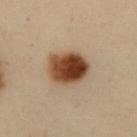Captured during whole-body skin photography for melanoma surveillance; the lesion was not biopsied. From the abdomen. A close-up tile cropped from a whole-body skin photograph, about 15 mm across. A female subject, aged around 50. Imaged with cross-polarized lighting. Measured at roughly 5 mm in maximum diameter. The lesion-visualizer software estimated a footprint of about 16 mm², a shape eccentricity near 0.55, and a shape-asymmetry score of about 0.1 (0 = symmetric). The analysis additionally found a lesion color around L≈38 a*≈17 b*≈28 in CIELAB, a lesion–skin lightness drop of about 16, and a normalized lesion–skin contrast near 13.5. And it measured a border-irregularity index near 1/10, internal color variation of about 9 on a 0–10 scale, and peripheral color asymmetry of about 3. The analysis additionally found a nevus-likeness score of about 100/100.